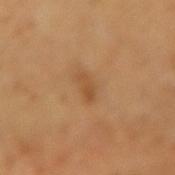The lesion was photographed on a routine skin check and not biopsied; there is no pathology result.